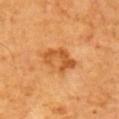Assessment: This lesion was catalogued during total-body skin photography and was not selected for biopsy. Context: The lesion's longest dimension is about 4 mm. The lesion is on the upper back. Cropped from a total-body skin-imaging series; the visible field is about 15 mm. The subject is a male approximately 65 years of age. Captured under cross-polarized illumination.